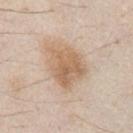site: chest
lesion_size:
  long_diameter_mm_approx: 5.5
image:
  source: total-body photography crop
  field_of_view_mm: 15
lighting: white-light
patient:
  sex: male
  age_approx: 80